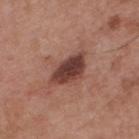Q: Was a biopsy performed?
A: catalogued during a skin exam; not biopsied
Q: Who is the patient?
A: male, approximately 55 years of age
Q: What is the imaging modality?
A: ~15 mm tile from a whole-body skin photo
Q: What did automated image analysis measure?
A: border irregularity of about 2.5 on a 0–10 scale, internal color variation of about 3.5 on a 0–10 scale, and a peripheral color-asymmetry measure near 1
Q: How was the tile lit?
A: white-light
Q: What is the anatomic site?
A: the upper back
Q: Lesion size?
A: ~5 mm (longest diameter)